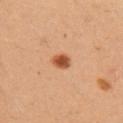Imaged during a routine full-body skin examination; the lesion was not biopsied and no histopathology is available.
About 2 mm across.
The lesion is on the right upper arm.
A 15 mm close-up tile from a total-body photography series done for melanoma screening.
Imaged with cross-polarized lighting.
A female subject roughly 35 years of age.